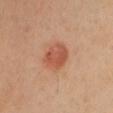follow-up: catalogued during a skin exam; not biopsied
tile lighting: cross-polarized
site: the chest
acquisition: ~15 mm tile from a whole-body skin photo
size: about 4 mm
patient: female, about 40 years old